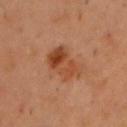{"biopsy_status": "not biopsied; imaged during a skin examination", "site": "chest", "lesion_size": {"long_diameter_mm_approx": 4.5}, "lighting": "cross-polarized", "patient": {"sex": "male", "age_approx": 55}, "image": {"source": "total-body photography crop", "field_of_view_mm": 15}, "automated_metrics": {"area_mm2_approx": 12.0, "eccentricity": 0.75, "shape_asymmetry": 0.3, "vs_skin_darker_L": 8.0, "vs_skin_contrast_norm": 7.5}}